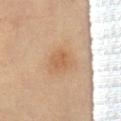The lesion was tiled from a total-body skin photograph and was not biopsied. On the abdomen. Cropped from a total-body skin-imaging series; the visible field is about 15 mm. The patient is a female about 60 years old. The tile uses cross-polarized illumination. The recorded lesion diameter is about 2.5 mm. Automated image analysis of the tile measured an average lesion color of about L≈61 a*≈21 b*≈37 (CIELAB). And it measured a border-irregularity rating of about 2/10 and radial color variation of about 0.5. The analysis additionally found a lesion-detection confidence of about 100/100.